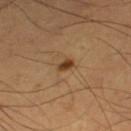| field | value |
|---|---|
| biopsy status | total-body-photography surveillance lesion; no biopsy |
| illumination | cross-polarized |
| automated lesion analysis | a lesion–skin lightness drop of about 12 and a lesion-to-skin contrast of about 10 (normalized; higher = more distinct) |
| diameter | ≈2 mm |
| patient | male, in their mid-50s |
| image | ~15 mm crop, total-body skin-cancer survey |
| location | the left thigh |